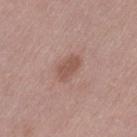No biopsy was performed on this lesion — it was imaged during a full skin examination and was not determined to be concerning. A 15 mm crop from a total-body photograph taken for skin-cancer surveillance. The recorded lesion diameter is about 3 mm. Located on the left thigh. A female patient aged approximately 40. This is a white-light tile.